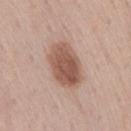• biopsy status · total-body-photography surveillance lesion; no biopsy
• lesion diameter · about 5.5 mm
• image · 15 mm crop, total-body photography
• subject · male, aged 73–77
• anatomic site · the back
• automated lesion analysis · a border-irregularity rating of about 1.5/10, a within-lesion color-variation index near 4.5/10, and a peripheral color-asymmetry measure near 1.5
• tile lighting · white-light illumination